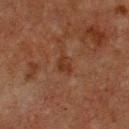{
  "biopsy_status": "not biopsied; imaged during a skin examination",
  "automated_metrics": {
    "color_variation_0_10": 1.5,
    "peripheral_color_asymmetry": 0.5
  },
  "site": "chest",
  "image": {
    "source": "total-body photography crop",
    "field_of_view_mm": 15
  },
  "lesion_size": {
    "long_diameter_mm_approx": 2.5
  },
  "lighting": "cross-polarized",
  "patient": {
    "sex": "male",
    "age_approx": 75
  }
}